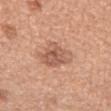biopsy status: catalogued during a skin exam; not biopsied
acquisition: ~15 mm tile from a whole-body skin photo
image-analysis metrics: a lesion area of about 10 mm², an eccentricity of roughly 0.6, and a shape-asymmetry score of about 0.25 (0 = symmetric); border irregularity of about 3 on a 0–10 scale and a peripheral color-asymmetry measure near 2
patient: male, in their mid- to late 60s
body site: the chest
lesion diameter: about 4 mm
illumination: white-light illumination